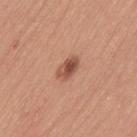Recorded during total-body skin imaging; not selected for excision or biopsy. The lesion is located on the left thigh. A 15 mm crop from a total-body photograph taken for skin-cancer surveillance. Approximately 3 mm at its widest. A female subject aged 28 to 32.